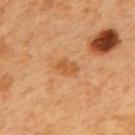The lesion was tiled from a total-body skin photograph and was not biopsied. A lesion tile, about 15 mm wide, cut from a 3D total-body photograph. The patient is a female approximately 40 years of age. On the mid back. Imaged with cross-polarized lighting. Automated image analysis of the tile measured an area of roughly 3.5 mm² and an eccentricity of roughly 0.8. It also reported a mean CIELAB color near L≈47 a*≈22 b*≈37, roughly 7 lightness units darker than nearby skin, and a lesion-to-skin contrast of about 6.5 (normalized; higher = more distinct). And it measured a border-irregularity rating of about 3/10 and a within-lesion color-variation index near 1.5/10. The analysis additionally found a classifier nevus-likeness of about 0/100 and a detector confidence of about 100 out of 100 that the crop contains a lesion. About 2.5 mm across.